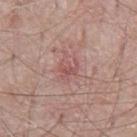Imaged during a routine full-body skin examination; the lesion was not biopsied and no histopathology is available. A male subject, approximately 65 years of age. A roughly 15 mm field-of-view crop from a total-body skin photograph. The lesion is located on the chest.